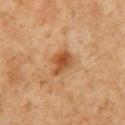patient = male, in their 60s; body site = the front of the torso; acquisition = ~15 mm tile from a whole-body skin photo.